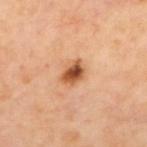Q: Is there a histopathology result?
A: no biopsy performed (imaged during a skin exam)
Q: How was this image acquired?
A: 15 mm crop, total-body photography
Q: Where on the body is the lesion?
A: the back
Q: Patient demographics?
A: male, in their mid-60s